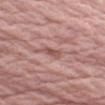Recorded during total-body skin imaging; not selected for excision or biopsy. Captured under white-light illumination. A lesion tile, about 15 mm wide, cut from a 3D total-body photograph. The patient is a male aged 73 to 77. The lesion is on the arm.